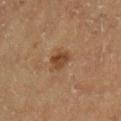notes: catalogued during a skin exam; not biopsied
tile lighting: cross-polarized
location: the left upper arm
patient: male, aged 58–62
TBP lesion metrics: border irregularity of about 2.5 on a 0–10 scale, internal color variation of about 3.5 on a 0–10 scale, and a peripheral color-asymmetry measure near 1
imaging modality: ~15 mm crop, total-body skin-cancer survey
size: about 2.5 mm